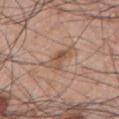| field | value |
|---|---|
| notes | no biopsy performed (imaged during a skin exam) |
| illumination | white-light illumination |
| imaging modality | ~15 mm crop, total-body skin-cancer survey |
| automated metrics | border irregularity of about 5.5 on a 0–10 scale, a color-variation rating of about 6/10, and radial color variation of about 2.5; a nevus-likeness score of about 0/100 and a lesion-detection confidence of about 100/100 |
| location | the front of the torso |
| subject | male, aged 68–72 |
| lesion size | ~4 mm (longest diameter) |